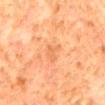The lesion was tiled from a total-body skin photograph and was not biopsied.